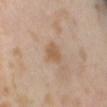Q: Is there a histopathology result?
A: no biopsy performed (imaged during a skin exam)
Q: Lesion location?
A: the back
Q: What are the patient's age and sex?
A: female, in their mid- to late 50s
Q: What did automated image analysis measure?
A: an area of roughly 4.5 mm² and an outline eccentricity of about 0.75 (0 = round, 1 = elongated); a mean CIELAB color near L≈57 a*≈17 b*≈32 and roughly 8 lightness units darker than nearby skin; a border-irregularity index near 2.5/10, a within-lesion color-variation index near 1.5/10, and peripheral color asymmetry of about 0.5; an automated nevus-likeness rating near 40 out of 100 and a detector confidence of about 100 out of 100 that the crop contains a lesion
Q: How was this image acquired?
A: total-body-photography crop, ~15 mm field of view